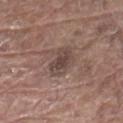Captured during whole-body skin photography for melanoma surveillance; the lesion was not biopsied. The lesion is on the chest. A lesion tile, about 15 mm wide, cut from a 3D total-body photograph. This is a white-light tile. A male subject, aged approximately 80. Longest diameter approximately 4 mm. The lesion-visualizer software estimated a footprint of about 7 mm² and an eccentricity of roughly 0.85. The analysis additionally found an average lesion color of about L≈44 a*≈16 b*≈20 (CIELAB) and a normalized border contrast of about 7.5. The analysis additionally found a border-irregularity index near 2/10 and a peripheral color-asymmetry measure near 1. It also reported a nevus-likeness score of about 0/100 and a detector confidence of about 100 out of 100 that the crop contains a lesion.